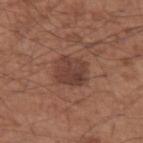Recorded during total-body skin imaging; not selected for excision or biopsy. A 15 mm crop from a total-body photograph taken for skin-cancer surveillance. About 3.5 mm across. This is a white-light tile. A male subject, roughly 55 years of age. The lesion is located on the left upper arm.